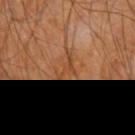image: ~15 mm tile from a whole-body skin photo | lighting: cross-polarized | diameter: ~3.5 mm (longest diameter) | patient: male, aged approximately 60 | site: the left upper arm.